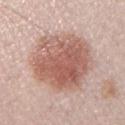{"biopsy_status": "not biopsied; imaged during a skin examination", "lighting": "white-light", "image": {"source": "total-body photography crop", "field_of_view_mm": 15}, "automated_metrics": {"area_mm2_approx": 41.0, "eccentricity": 0.3, "cielab_L": 59, "cielab_a": 22, "cielab_b": 26, "vs_skin_darker_L": 13.0, "border_irregularity_0_10": 1.5, "color_variation_0_10": 6.0, "peripheral_color_asymmetry": 2.0}, "patient": {"sex": "male", "age_approx": 30}}